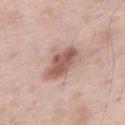Captured during whole-body skin photography for melanoma surveillance; the lesion was not biopsied. An algorithmic analysis of the crop reported an area of roughly 10 mm², a shape eccentricity near 0.85, and a shape-asymmetry score of about 0.2 (0 = symmetric). The software also gave a classifier nevus-likeness of about 45/100. Captured under white-light illumination. From the right thigh. A roughly 15 mm field-of-view crop from a total-body skin photograph. The patient is a male aged approximately 55. The lesion's longest dimension is about 5 mm.